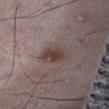| feature | finding |
|---|---|
| follow-up | imaged on a skin check; not biopsied |
| imaging modality | total-body-photography crop, ~15 mm field of view |
| patient | male, aged around 50 |
| size | ~4 mm (longest diameter) |
| automated lesion analysis | a lesion area of about 7 mm², an outline eccentricity of about 0.8 (0 = round, 1 = elongated), and a shape-asymmetry score of about 0.2 (0 = symmetric) |
| location | the right thigh |